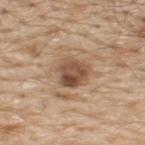Clinical impression:
The lesion was photographed on a routine skin check and not biopsied; there is no pathology result.
Clinical summary:
On the back. This is a white-light tile. A male patient, aged 68 to 72. A 15 mm crop from a total-body photograph taken for skin-cancer surveillance. Automated tile analysis of the lesion measured a border-irregularity index near 2/10, a within-lesion color-variation index near 6.5/10, and peripheral color asymmetry of about 2.5. And it measured an automated nevus-likeness rating near 35 out of 100 and lesion-presence confidence of about 100/100.